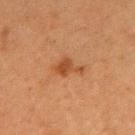biopsy status: total-body-photography surveillance lesion; no biopsy
imaging modality: ~15 mm crop, total-body skin-cancer survey
body site: the left upper arm
automated metrics: an area of roughly 4.5 mm², an outline eccentricity of about 0.85 (0 = round, 1 = elongated), and two-axis asymmetry of about 0.4; a lesion color around L≈39 a*≈22 b*≈33 in CIELAB, about 8 CIELAB-L* units darker than the surrounding skin, and a normalized border contrast of about 7.5; a border-irregularity rating of about 4.5/10, a within-lesion color-variation index near 1.5/10, and a peripheral color-asymmetry measure near 0.5; a classifier nevus-likeness of about 75/100 and lesion-presence confidence of about 100/100
patient: female, aged 58 to 62
lesion size: ≈3.5 mm
tile lighting: cross-polarized